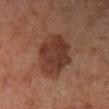Captured during whole-body skin photography for melanoma surveillance; the lesion was not biopsied.
The lesion is on the right lower leg.
A 15 mm close-up extracted from a 3D total-body photography capture.
Longest diameter approximately 5.5 mm.
Imaged with cross-polarized lighting.
The subject is a male aged 68 to 72.